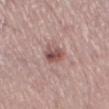{"biopsy_status": "not biopsied; imaged during a skin examination", "automated_metrics": {"nevus_likeness_0_100": 85, "lesion_detection_confidence_0_100": 100}, "patient": {"sex": "male", "age_approx": 60}, "site": "left thigh", "image": {"source": "total-body photography crop", "field_of_view_mm": 15}, "lighting": "white-light"}